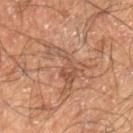From the right lower leg. A 15 mm crop from a total-body photograph taken for skin-cancer surveillance. Captured under cross-polarized illumination. A male subject roughly 60 years of age. Measured at roughly 6 mm in maximum diameter. Automated tile analysis of the lesion measured a mean CIELAB color near L≈48 a*≈19 b*≈29 and a lesion-to-skin contrast of about 5.5 (normalized; higher = more distinct).